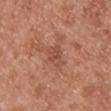Impression: The lesion was tiled from a total-body skin photograph and was not biopsied. Background: The patient is a female aged approximately 40. The lesion is located on the chest. Imaged with white-light lighting. A 15 mm close-up extracted from a 3D total-body photography capture.